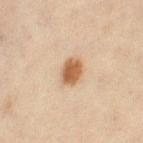Impression: Recorded during total-body skin imaging; not selected for excision or biopsy. Background: A close-up tile cropped from a whole-body skin photograph, about 15 mm across. The lesion is located on the right thigh. A female patient roughly 45 years of age. Captured under cross-polarized illumination.